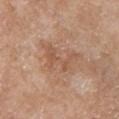{"biopsy_status": "not biopsied; imaged during a skin examination", "patient": {"sex": "female", "age_approx": 70}, "site": "left upper arm", "lesion_size": {"long_diameter_mm_approx": 5.0}, "image": {"source": "total-body photography crop", "field_of_view_mm": 15}, "automated_metrics": {"area_mm2_approx": 10.0, "eccentricity": 0.8, "shape_asymmetry": 0.5, "border_irregularity_0_10": 8.5, "color_variation_0_10": 3.0, "peripheral_color_asymmetry": 1.0}}